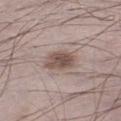Assessment:
Part of a total-body skin-imaging series; this lesion was reviewed on a skin check and was not flagged for biopsy.
Acquisition and patient details:
The patient is a male aged approximately 70. The lesion is located on the left lower leg. The recorded lesion diameter is about 4 mm. A lesion tile, about 15 mm wide, cut from a 3D total-body photograph. Captured under white-light illumination. The total-body-photography lesion software estimated a lesion area of about 9 mm², an eccentricity of roughly 0.7, and a shape-asymmetry score of about 0.15 (0 = symmetric). The software also gave a nevus-likeness score of about 80/100.